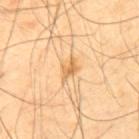biopsy status=catalogued during a skin exam; not biopsied
subject=male, aged 38 to 42
imaging modality=~15 mm crop, total-body skin-cancer survey
site=the upper back